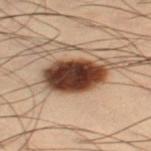Imaged during a routine full-body skin examination; the lesion was not biopsied and no histopathology is available. About 6 mm across. Automated image analysis of the tile measured a mean CIELAB color near L≈33 a*≈16 b*≈24 and a lesion–skin lightness drop of about 20. It also reported a classifier nevus-likeness of about 95/100 and a lesion-detection confidence of about 100/100. A 15 mm close-up extracted from a 3D total-body photography capture. The patient is a male aged around 55. Imaged with cross-polarized lighting. From the right thigh.